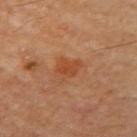The lesion was tiled from a total-body skin photograph and was not biopsied.
On the right upper arm.
A 15 mm close-up extracted from a 3D total-body photography capture.
The total-body-photography lesion software estimated a footprint of about 5 mm² and two-axis asymmetry of about 0.25.